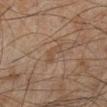Assessment:
Part of a total-body skin-imaging series; this lesion was reviewed on a skin check and was not flagged for biopsy.
Context:
A 15 mm close-up tile from a total-body photography series done for melanoma screening. The lesion is located on the leg. The subject is a male aged 43–47.